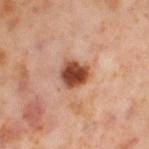Captured during whole-body skin photography for melanoma surveillance; the lesion was not biopsied. Captured under cross-polarized illumination. Automated tile analysis of the lesion measured a lesion color around L≈48 a*≈26 b*≈33 in CIELAB. On the leg. Longest diameter approximately 3 mm. A close-up tile cropped from a whole-body skin photograph, about 15 mm across. A female patient, in their mid- to late 50s.